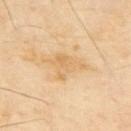follow-up=no biopsy performed (imaged during a skin exam); patient=male, approximately 65 years of age; image source=~15 mm crop, total-body skin-cancer survey; automated metrics=a footprint of about 8.5 mm², an eccentricity of roughly 0.7, and two-axis asymmetry of about 0.5; body site=the back; lighting=cross-polarized illumination.